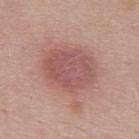| feature | finding |
|---|---|
| lesion diameter | ~6 mm (longest diameter) |
| image source | total-body-photography crop, ~15 mm field of view |
| location | the upper back |
| subject | male, aged around 25 |
| illumination | white-light illumination |